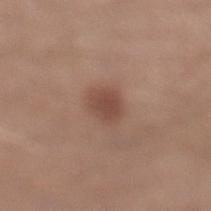Assessment:
The lesion was tiled from a total-body skin photograph and was not biopsied.
Acquisition and patient details:
Imaged with white-light lighting. The lesion is on the left lower leg. This image is a 15 mm lesion crop taken from a total-body photograph. A male patient about 30 years old.